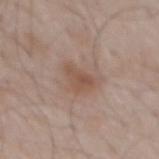notes: total-body-photography surveillance lesion; no biopsy | subject: male, roughly 55 years of age | tile lighting: white-light illumination | lesion size: ≈3.5 mm | automated metrics: a mean CIELAB color near L≈52 a*≈18 b*≈26, a lesion–skin lightness drop of about 8, and a lesion-to-skin contrast of about 6.5 (normalized; higher = more distinct); a border-irregularity rating of about 4/10, a within-lesion color-variation index near 3/10, and a peripheral color-asymmetry measure near 1 | image source: ~15 mm tile from a whole-body skin photo | location: the mid back.